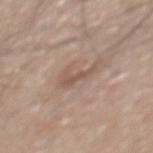This is a white-light tile.
A close-up tile cropped from a whole-body skin photograph, about 15 mm across.
The patient is a male in their mid-60s.
The lesion is located on the mid back.